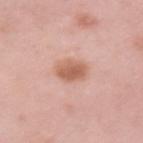patient:
  sex: female
  age_approx: 50
lesion_size:
  long_diameter_mm_approx: 3.5
automated_metrics:
  area_mm2_approx: 7.0
  eccentricity: 0.55
  border_irregularity_0_10: 1.5
  peripheral_color_asymmetry: 1.0
  nevus_likeness_0_100: 85
  lesion_detection_confidence_0_100: 100
image:
  source: total-body photography crop
  field_of_view_mm: 15
site: left upper arm